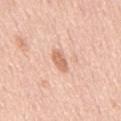| feature | finding |
|---|---|
| follow-up | no biopsy performed (imaged during a skin exam) |
| tile lighting | white-light |
| subject | male, aged approximately 65 |
| anatomic site | the mid back |
| TBP lesion metrics | a lesion color around L≈67 a*≈23 b*≈32 in CIELAB, about 11 CIELAB-L* units darker than the surrounding skin, and a normalized border contrast of about 7; a border-irregularity rating of about 2.5/10, a color-variation rating of about 2/10, and a peripheral color-asymmetry measure near 0.5 |
| imaging modality | ~15 mm tile from a whole-body skin photo |